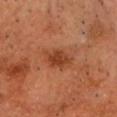follow-up: no biopsy performed (imaged during a skin exam)
subject: male, aged approximately 70
automated lesion analysis: an area of roughly 7.5 mm², an outline eccentricity of about 0.8 (0 = round, 1 = elongated), and a shape-asymmetry score of about 0.15 (0 = symmetric); a border-irregularity rating of about 2/10 and internal color variation of about 2.5 on a 0–10 scale; a classifier nevus-likeness of about 70/100 and a lesion-detection confidence of about 100/100
lesion diameter: ~4.5 mm (longest diameter)
body site: the front of the torso
image: 15 mm crop, total-body photography
tile lighting: cross-polarized illumination